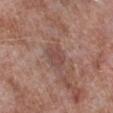Recorded during total-body skin imaging; not selected for excision or biopsy.
A male patient, aged approximately 55.
Automated tile analysis of the lesion measured a lesion area of about 5.5 mm² and an eccentricity of roughly 0.65. It also reported a mean CIELAB color near L≈47 a*≈20 b*≈23, a lesion–skin lightness drop of about 7, and a normalized border contrast of about 5.5. The software also gave a border-irregularity index near 3/10 and peripheral color asymmetry of about 0.5. And it measured a classifier nevus-likeness of about 0/100.
A 15 mm close-up extracted from a 3D total-body photography capture.
The lesion is located on the right lower leg.
Longest diameter approximately 3 mm.